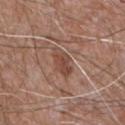imaging modality: ~15 mm crop, total-body skin-cancer survey | illumination: white-light illumination | lesion size: ≈4.5 mm | automated metrics: an outline eccentricity of about 0.85 (0 = round, 1 = elongated) and a shape-asymmetry score of about 0.25 (0 = symmetric); a mean CIELAB color near L≈47 a*≈21 b*≈26 and a lesion-to-skin contrast of about 6.5 (normalized; higher = more distinct); a classifier nevus-likeness of about 5/100 and a detector confidence of about 95 out of 100 that the crop contains a lesion | body site: the upper back | patient: male, aged around 60.